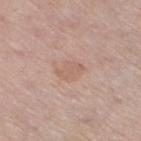follow-up: total-body-photography surveillance lesion; no biopsy
image: total-body-photography crop, ~15 mm field of view
body site: the right thigh
image-analysis metrics: a lesion area of about 6 mm², an outline eccentricity of about 0.75 (0 = round, 1 = elongated), and a symmetry-axis asymmetry near 0.2; a lesion color around L≈61 a*≈19 b*≈27 in CIELAB, a lesion–skin lightness drop of about 6, and a normalized lesion–skin contrast near 4.5; a border-irregularity index near 2/10 and a within-lesion color-variation index near 3/10; a classifier nevus-likeness of about 0/100 and lesion-presence confidence of about 100/100
patient: male, aged approximately 70
size: about 3.5 mm
lighting: white-light illumination